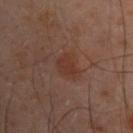<lesion>
  <biopsy_status>not biopsied; imaged during a skin examination</biopsy_status>
  <image>
    <source>total-body photography crop</source>
    <field_of_view_mm>15</field_of_view_mm>
  </image>
  <site>left arm</site>
  <lesion_size>
    <long_diameter_mm_approx>2.5</long_diameter_mm_approx>
  </lesion_size>
  <automated_metrics>
    <eccentricity>0.6</eccentricity>
    <shape_asymmetry>0.3</shape_asymmetry>
    <border_irregularity_0_10>2.5</border_irregularity_0_10>
    <peripheral_color_asymmetry>0.5</peripheral_color_asymmetry>
    <nevus_likeness_0_100>20</nevus_likeness_0_100>
    <lesion_detection_confidence_0_100>100</lesion_detection_confidence_0_100>
  </automated_metrics>
  <patient>
    <sex>male</sex>
    <age_approx>50</age_approx>
  </patient>
</lesion>